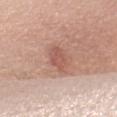| feature | finding |
|---|---|
| workup | catalogued during a skin exam; not biopsied |
| subject | female, in their 60s |
| illumination | white-light |
| lesion diameter | ≈3.5 mm |
| image | total-body-photography crop, ~15 mm field of view |
| TBP lesion metrics | a shape eccentricity near 0.75 and a symmetry-axis asymmetry near 0.15; a classifier nevus-likeness of about 10/100 and lesion-presence confidence of about 100/100 |
| site | the left upper arm |